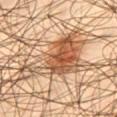Assessment:
Recorded during total-body skin imaging; not selected for excision or biopsy.
Clinical summary:
A male patient, aged approximately 45. Approximately 9 mm at its widest. An algorithmic analysis of the crop reported a footprint of about 25 mm², an outline eccentricity of about 0.8 (0 = round, 1 = elongated), and a symmetry-axis asymmetry near 0.65. The software also gave a mean CIELAB color near L≈55 a*≈21 b*≈35, a lesion–skin lightness drop of about 14, and a normalized lesion–skin contrast near 9. The analysis additionally found a border-irregularity rating of about 8/10, a color-variation rating of about 8/10, and a peripheral color-asymmetry measure near 2.5. Cropped from a total-body skin-imaging series; the visible field is about 15 mm. Captured under cross-polarized illumination. From the right thigh.